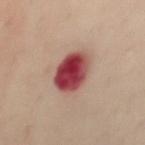Case summary:
- follow-up: total-body-photography surveillance lesion; no biopsy
- image-analysis metrics: a lesion color around L≈45 a*≈34 b*≈23 in CIELAB, roughly 20 lightness units darker than nearby skin, and a normalized border contrast of about 14; a border-irregularity index near 1.5/10 and internal color variation of about 8.5 on a 0–10 scale; an automated nevus-likeness rating near 0 out of 100 and a lesion-detection confidence of about 100/100
- lighting: cross-polarized
- body site: the chest
- acquisition: 15 mm crop, total-body photography
- diameter: ≈4 mm
- patient: female, about 55 years old